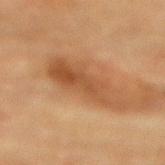Q: Was a biopsy performed?
A: imaged on a skin check; not biopsied
Q: What lighting was used for the tile?
A: cross-polarized illumination
Q: Lesion location?
A: the mid back
Q: What kind of image is this?
A: 15 mm crop, total-body photography
Q: What is the lesion's diameter?
A: ≈7.5 mm
Q: Who is the patient?
A: female, in their 80s